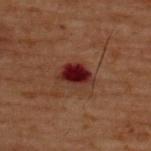  biopsy_status: not biopsied; imaged during a skin examination
  patient:
    sex: male
    age_approx: 60
  site: upper back
  image:
    source: total-body photography crop
    field_of_view_mm: 15